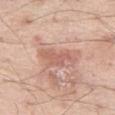  biopsy_status: not biopsied; imaged during a skin examination
  patient:
    sex: male
    age_approx: 55
  image:
    source: total-body photography crop
    field_of_view_mm: 15
  site: leg